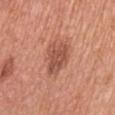Acquisition and patient details:
A female subject in their mid- to late 60s. The lesion is on the left upper arm. This image is a 15 mm lesion crop taken from a total-body photograph. The tile uses white-light illumination. Measured at roughly 4 mm in maximum diameter. The total-body-photography lesion software estimated a lesion area of about 10 mm², a shape eccentricity near 0.65, and two-axis asymmetry of about 0.25. The analysis additionally found a lesion color around L≈52 a*≈26 b*≈30 in CIELAB, roughly 11 lightness units darker than nearby skin, and a normalized lesion–skin contrast near 7. The software also gave a border-irregularity index near 3/10 and peripheral color asymmetry of about 1. And it measured a classifier nevus-likeness of about 45/100 and lesion-presence confidence of about 100/100.